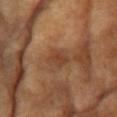Background:
A 15 mm close-up tile from a total-body photography series done for melanoma screening. The total-body-photography lesion software estimated a lesion area of about 5.5 mm², a shape eccentricity near 0.6, and a symmetry-axis asymmetry near 0.25. And it measured an average lesion color of about L≈42 a*≈21 b*≈31 (CIELAB), roughly 8 lightness units darker than nearby skin, and a lesion-to-skin contrast of about 6 (normalized; higher = more distinct). The analysis additionally found a border-irregularity index near 2/10. Located on the head or neck. Measured at roughly 3 mm in maximum diameter. A female patient about 75 years old.